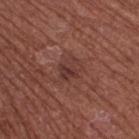| key | value |
|---|---|
| biopsy status | imaged on a skin check; not biopsied |
| illumination | white-light |
| body site | the right thigh |
| patient | male, aged 63–67 |
| imaging modality | ~15 mm tile from a whole-body skin photo |
| lesion size | about 3 mm |
| image-analysis metrics | a border-irregularity index near 3/10, a color-variation rating of about 5/10, and radial color variation of about 2; an automated nevus-likeness rating near 0 out of 100 |